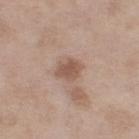This lesion was catalogued during total-body skin photography and was not selected for biopsy. A lesion tile, about 15 mm wide, cut from a 3D total-body photograph. A female patient in their mid- to late 50s. From the leg. The recorded lesion diameter is about 2.5 mm. Captured under white-light illumination.